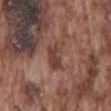Case summary:
* follow-up: total-body-photography surveillance lesion; no biopsy
* site: the mid back
* acquisition: 15 mm crop, total-body photography
* subject: male, aged 73–77